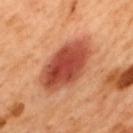The lesion was tiled from a total-body skin photograph and was not biopsied. Imaged with cross-polarized lighting. The subject is a male roughly 50 years of age. A lesion tile, about 15 mm wide, cut from a 3D total-body photograph. The lesion is on the mid back.